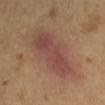follow-up = catalogued during a skin exam; not biopsied | image source = ~15 mm tile from a whole-body skin photo | patient = female, aged approximately 40 | anatomic site = the left forearm.